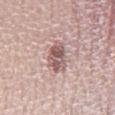| field | value |
|---|---|
| biopsy status | catalogued during a skin exam; not biopsied |
| site | the left lower leg |
| subject | female, aged 28 to 32 |
| size | ~4.5 mm (longest diameter) |
| lighting | white-light |
| imaging modality | 15 mm crop, total-body photography |